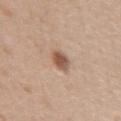Assessment: The lesion was tiled from a total-body skin photograph and was not biopsied. Image and clinical context: An algorithmic analysis of the crop reported an area of roughly 4.5 mm², an outline eccentricity of about 0.6 (0 = round, 1 = elongated), and a symmetry-axis asymmetry near 0.2. And it measured border irregularity of about 1.5 on a 0–10 scale, a color-variation rating of about 3.5/10, and peripheral color asymmetry of about 1. The analysis additionally found an automated nevus-likeness rating near 90 out of 100 and lesion-presence confidence of about 100/100. The lesion is on the right upper arm. Measured at roughly 2.5 mm in maximum diameter. A female subject, aged 28 to 32. A 15 mm close-up extracted from a 3D total-body photography capture.